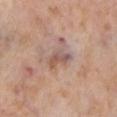notes: total-body-photography surveillance lesion; no biopsy | acquisition: ~15 mm tile from a whole-body skin photo | lesion diameter: ~4 mm (longest diameter) | lighting: cross-polarized | location: the leg | subject: female, aged 53–57.